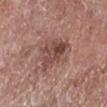– biopsy status — catalogued during a skin exam; not biopsied
– automated metrics — a mean CIELAB color near L≈46 a*≈21 b*≈23; a classifier nevus-likeness of about 10/100 and a detector confidence of about 100 out of 100 that the crop contains a lesion
– subject — male, aged 73 to 77
– acquisition — ~15 mm tile from a whole-body skin photo
– diameter — about 4.5 mm
– anatomic site — the right lower leg
– lighting — white-light illumination